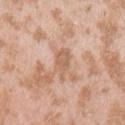Assessment: Part of a total-body skin-imaging series; this lesion was reviewed on a skin check and was not flagged for biopsy. Clinical summary: The subject is a female about 25 years old. Measured at roughly 4 mm in maximum diameter. A lesion tile, about 15 mm wide, cut from a 3D total-body photograph. The lesion is located on the left upper arm. Imaged with white-light lighting.